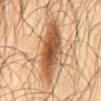Clinical impression: Recorded during total-body skin imaging; not selected for excision or biopsy. Acquisition and patient details: The lesion's longest dimension is about 8 mm. Located on the mid back. The patient is a male in their mid- to late 60s. This is a cross-polarized tile. This image is a 15 mm lesion crop taken from a total-body photograph. The lesion-visualizer software estimated a mean CIELAB color near L≈52 a*≈21 b*≈36, about 16 CIELAB-L* units darker than the surrounding skin, and a normalized lesion–skin contrast near 10.5. The software also gave border irregularity of about 3.5 on a 0–10 scale, a color-variation rating of about 7.5/10, and peripheral color asymmetry of about 2. The analysis additionally found a nevus-likeness score of about 100/100 and a detector confidence of about 100 out of 100 that the crop contains a lesion.